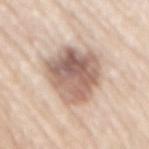{"biopsy_status": "not biopsied; imaged during a skin examination", "image": {"source": "total-body photography crop", "field_of_view_mm": 15}, "site": "lower back", "lighting": "white-light", "patient": {"sex": "male", "age_approx": 80}, "lesion_size": {"long_diameter_mm_approx": 7.0}}